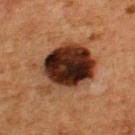Assessment: The lesion was tiled from a total-body skin photograph and was not biopsied. Context: A 15 mm close-up tile from a total-body photography series done for melanoma screening. The patient is a male about 65 years old. Imaged with cross-polarized lighting. The lesion is located on the upper back.